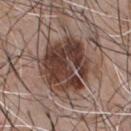* workup: imaged on a skin check; not biopsied
* lighting: white-light
* size: about 6.5 mm
* image source: ~15 mm tile from a whole-body skin photo
* body site: the chest
* subject: male, approximately 45 years of age